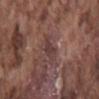| field | value |
|---|---|
| biopsy status | no biopsy performed (imaged during a skin exam) |
| illumination | white-light |
| subject | male, in their mid- to late 70s |
| image | ~15 mm crop, total-body skin-cancer survey |
| lesion diameter | about 3 mm |
| automated metrics | a lesion area of about 4 mm², an outline eccentricity of about 0.85 (0 = round, 1 = elongated), and a shape-asymmetry score of about 0.35 (0 = symmetric); border irregularity of about 3 on a 0–10 scale and a within-lesion color-variation index near 1.5/10 |
| body site | the mid back |